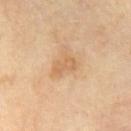Captured during whole-body skin photography for melanoma surveillance; the lesion was not biopsied.
The lesion is on the chest.
The patient is a male approximately 70 years of age.
A 15 mm crop from a total-body photograph taken for skin-cancer surveillance.